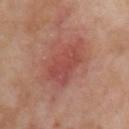Captured during whole-body skin photography for melanoma surveillance; the lesion was not biopsied. A female subject, in their 50s. A close-up tile cropped from a whole-body skin photograph, about 15 mm across. The lesion is on the left upper arm.